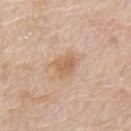Clinical impression: No biopsy was performed on this lesion — it was imaged during a full skin examination and was not determined to be concerning. Clinical summary: From the mid back. About 3 mm across. This is a white-light tile. A lesion tile, about 15 mm wide, cut from a 3D total-body photograph. The patient is a male approximately 65 years of age.